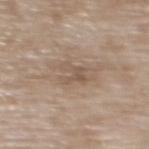Clinical summary: Longest diameter approximately 3.5 mm. Cropped from a whole-body photographic skin survey; the tile spans about 15 mm. The patient is a female approximately 75 years of age. Captured under white-light illumination. The lesion is located on the back.